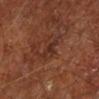  biopsy_status: not biopsied; imaged during a skin examination
  image:
    source: total-body photography crop
    field_of_view_mm: 15
  site: left lower leg
  patient:
    sex: male
    age_approx: 65
  lesion_size:
    long_diameter_mm_approx: 3.0
  automated_metrics:
    border_irregularity_0_10: 4.5
    color_variation_0_10: 0.0
    peripheral_color_asymmetry: 0.0
  lighting: cross-polarized